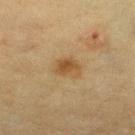No biopsy was performed on this lesion — it was imaged during a full skin examination and was not determined to be concerning. Measured at roughly 3 mm in maximum diameter. A female subject, aged around 55. Automated image analysis of the tile measured a lesion area of about 5.5 mm², a shape eccentricity near 0.7, and two-axis asymmetry of about 0.25. The analysis additionally found border irregularity of about 2 on a 0–10 scale, a color-variation rating of about 3/10, and a peripheral color-asymmetry measure near 1. And it measured a classifier nevus-likeness of about 90/100. Located on the left lower leg. A lesion tile, about 15 mm wide, cut from a 3D total-body photograph. Captured under cross-polarized illumination.